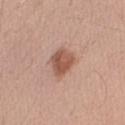The lesion was photographed on a routine skin check and not biopsied; there is no pathology result. Imaged with white-light lighting. The lesion-visualizer software estimated a mean CIELAB color near L≈54 a*≈22 b*≈29, roughly 12 lightness units darker than nearby skin, and a lesion-to-skin contrast of about 8.5 (normalized; higher = more distinct). It also reported an automated nevus-likeness rating near 95 out of 100 and a detector confidence of about 100 out of 100 that the crop contains a lesion. The lesion's longest dimension is about 3.5 mm. From the arm. A male subject aged 38–42. A lesion tile, about 15 mm wide, cut from a 3D total-body photograph.